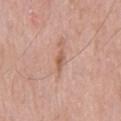Findings:
* workup — imaged on a skin check; not biopsied
* imaging modality — ~15 mm tile from a whole-body skin photo
* subject — male, aged 63–67
* TBP lesion metrics — a lesion area of about 3 mm², a shape eccentricity near 0.9, and a shape-asymmetry score of about 0.35 (0 = symmetric); a detector confidence of about 100 out of 100 that the crop contains a lesion
* lesion diameter — ~2.5 mm (longest diameter)
* location — the front of the torso